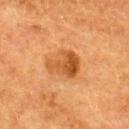{
  "biopsy_status": "not biopsied; imaged during a skin examination",
  "site": "back",
  "automated_metrics": {
    "cielab_L": 45,
    "cielab_a": 23,
    "cielab_b": 38,
    "vs_skin_darker_L": 10.0,
    "border_irregularity_0_10": 3.0,
    "color_variation_0_10": 6.0,
    "peripheral_color_asymmetry": 2.5,
    "nevus_likeness_0_100": 75,
    "lesion_detection_confidence_0_100": 100
  },
  "lesion_size": {
    "long_diameter_mm_approx": 4.0
  },
  "image": {
    "source": "total-body photography crop",
    "field_of_view_mm": 15
  },
  "patient": {
    "sex": "female",
    "age_approx": 55
  },
  "lighting": "cross-polarized"
}